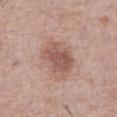| field | value |
|---|---|
| workup | no biopsy performed (imaged during a skin exam) |
| imaging modality | total-body-photography crop, ~15 mm field of view |
| lesion size | ~5 mm (longest diameter) |
| patient | male, about 75 years old |
| illumination | white-light illumination |
| anatomic site | the front of the torso |
| automated metrics | a lesion area of about 12 mm², an eccentricity of roughly 0.7, and a symmetry-axis asymmetry near 0.25; an average lesion color of about L≈54 a*≈22 b*≈25 (CIELAB), a lesion–skin lightness drop of about 11, and a normalized lesion–skin contrast near 7.5 |